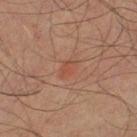{
  "biopsy_status": "not biopsied; imaged during a skin examination",
  "patient": {
    "sex": "male",
    "age_approx": 60
  },
  "automated_metrics": {
    "vs_skin_darker_L": 5.0,
    "vs_skin_contrast_norm": 4.5,
    "border_irregularity_0_10": 2.5,
    "color_variation_0_10": 1.5,
    "peripheral_color_asymmetry": 0.5
  },
  "lesion_size": {
    "long_diameter_mm_approx": 2.5
  },
  "site": "leg",
  "lighting": "cross-polarized",
  "image": {
    "source": "total-body photography crop",
    "field_of_view_mm": 15
  }
}